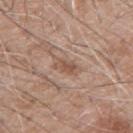Imaged during a routine full-body skin examination; the lesion was not biopsied and no histopathology is available. Imaged with white-light lighting. From the right upper arm. A male patient, aged approximately 60. A 15 mm close-up extracted from a 3D total-body photography capture. The lesion's longest dimension is about 3 mm.